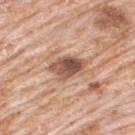Background: The lesion-visualizer software estimated an area of roughly 9 mm², an eccentricity of roughly 0.8, and two-axis asymmetry of about 0.25. The software also gave border irregularity of about 3 on a 0–10 scale, a color-variation rating of about 8/10, and radial color variation of about 3. Cropped from a whole-body photographic skin survey; the tile spans about 15 mm. The lesion is on the upper back. Approximately 4.5 mm at its widest. A male subject, in their 80s.